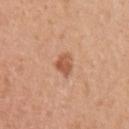Imaged during a routine full-body skin examination; the lesion was not biopsied and no histopathology is available. A female patient, in their 50s. Imaged with white-light lighting. The lesion is located on the left upper arm. Automated tile analysis of the lesion measured a lesion area of about 4.5 mm², an outline eccentricity of about 0.4 (0 = round, 1 = elongated), and a shape-asymmetry score of about 0.25 (0 = symmetric). And it measured a lesion-to-skin contrast of about 7.5 (normalized; higher = more distinct). And it measured an automated nevus-likeness rating near 70 out of 100 and a lesion-detection confidence of about 100/100. About 2.5 mm across. This image is a 15 mm lesion crop taken from a total-body photograph.